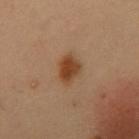Imaged during a routine full-body skin examination; the lesion was not biopsied and no histopathology is available. Imaged with cross-polarized lighting. Cropped from a total-body skin-imaging series; the visible field is about 15 mm. A male subject in their mid-50s. On the chest. The recorded lesion diameter is about 4 mm.